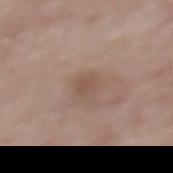notes = catalogued during a skin exam; not biopsied
tile lighting = white-light
imaging modality = total-body-photography crop, ~15 mm field of view
subject = male, aged 83 or over
body site = the upper back
lesion diameter = ~3 mm (longest diameter)